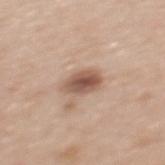Imaged during a routine full-body skin examination; the lesion was not biopsied and no histopathology is available.
Located on the back.
A 15 mm crop from a total-body photograph taken for skin-cancer surveillance.
The patient is a female approximately 45 years of age.